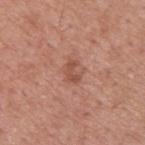Clinical impression:
Captured during whole-body skin photography for melanoma surveillance; the lesion was not biopsied.
Acquisition and patient details:
Automated tile analysis of the lesion measured a lesion area of about 3.5 mm², an outline eccentricity of about 0.75 (0 = round, 1 = elongated), and a shape-asymmetry score of about 0.4 (0 = symmetric). The analysis additionally found a lesion color around L≈51 a*≈24 b*≈29 in CIELAB, about 9 CIELAB-L* units darker than the surrounding skin, and a lesion-to-skin contrast of about 6.5 (normalized; higher = more distinct). It also reported a border-irregularity rating of about 4/10, internal color variation of about 1.5 on a 0–10 scale, and a peripheral color-asymmetry measure near 0.5. And it measured an automated nevus-likeness rating near 0 out of 100 and a detector confidence of about 100 out of 100 that the crop contains a lesion. The lesion's longest dimension is about 2.5 mm. The tile uses white-light illumination. A 15 mm crop from a total-body photograph taken for skin-cancer surveillance. From the upper back. The patient is a male approximately 55 years of age.